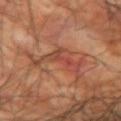Clinical impression:
The lesion was photographed on a routine skin check and not biopsied; there is no pathology result.
Acquisition and patient details:
About 5.5 mm across. Cropped from a whole-body photographic skin survey; the tile spans about 15 mm. The lesion is on the left upper arm. The lesion-visualizer software estimated an average lesion color of about L≈45 a*≈26 b*≈30 (CIELAB) and a normalized border contrast of about 6. And it measured border irregularity of about 10 on a 0–10 scale, a color-variation rating of about 6/10, and peripheral color asymmetry of about 2.5. It also reported a nevus-likeness score of about 0/100 and a detector confidence of about 55 out of 100 that the crop contains a lesion. Imaged with cross-polarized lighting. A male patient aged approximately 60.